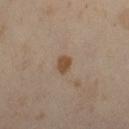follow-up: total-body-photography surveillance lesion; no biopsy | image: total-body-photography crop, ~15 mm field of view | subject: female, aged 48 to 52 | lesion size: ~2 mm (longest diameter) | lighting: cross-polarized illumination | site: the left forearm.